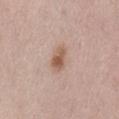No biopsy was performed on this lesion — it was imaged during a full skin examination and was not determined to be concerning.
A 15 mm crop from a total-body photograph taken for skin-cancer surveillance.
The patient is a male aged around 75.
The lesion is on the lower back.